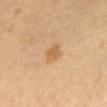The lesion is on the abdomen.
A male patient, approximately 65 years of age.
Cropped from a whole-body photographic skin survey; the tile spans about 15 mm.
About 2.5 mm across.